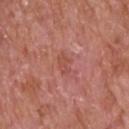The lesion was photographed on a routine skin check and not biopsied; there is no pathology result.
The subject is a male aged approximately 65.
The lesion's longest dimension is about 2.5 mm.
A close-up tile cropped from a whole-body skin photograph, about 15 mm across.
This is a white-light tile.
Automated tile analysis of the lesion measured a lesion area of about 3.5 mm², a shape eccentricity near 0.75, and a shape-asymmetry score of about 0.3 (0 = symmetric). And it measured a mean CIELAB color near L≈51 a*≈28 b*≈29, about 6 CIELAB-L* units darker than the surrounding skin, and a normalized lesion–skin contrast near 5. And it measured a border-irregularity index near 3.5/10, internal color variation of about 1 on a 0–10 scale, and peripheral color asymmetry of about 0. The software also gave an automated nevus-likeness rating near 0 out of 100 and a detector confidence of about 100 out of 100 that the crop contains a lesion.
From the upper back.